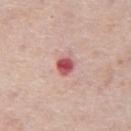Clinical impression:
Captured during whole-body skin photography for melanoma surveillance; the lesion was not biopsied.
Image and clinical context:
The patient is a male in their mid- to late 70s. Cropped from a whole-body photographic skin survey; the tile spans about 15 mm. Imaged with white-light lighting. The total-body-photography lesion software estimated an average lesion color of about L≈55 a*≈32 b*≈23 (CIELAB). The software also gave a classifier nevus-likeness of about 0/100 and a lesion-detection confidence of about 100/100. The lesion's longest dimension is about 2.5 mm. Located on the abdomen.